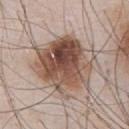This lesion was catalogued during total-body skin photography and was not selected for biopsy.
On the chest.
A male subject, approximately 55 years of age.
Automated tile analysis of the lesion measured an eccentricity of roughly 0.25 and a shape-asymmetry score of about 0.15 (0 = symmetric). It also reported an automated nevus-likeness rating near 90 out of 100 and a detector confidence of about 100 out of 100 that the crop contains a lesion.
A region of skin cropped from a whole-body photographic capture, roughly 15 mm wide.
Approximately 8 mm at its widest.
This is a white-light tile.